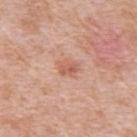Clinical impression:
This lesion was catalogued during total-body skin photography and was not selected for biopsy.
Acquisition and patient details:
This is a white-light tile. A 15 mm close-up tile from a total-body photography series done for melanoma screening. On the upper back. The patient is a male aged around 50.